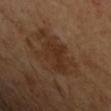About 6 mm across. The lesion-visualizer software estimated a lesion area of about 18 mm², an eccentricity of roughly 0.75, and a symmetry-axis asymmetry near 0.4. A male patient aged approximately 45. From the left upper arm. Cropped from a total-body skin-imaging series; the visible field is about 15 mm. This is a cross-polarized tile.